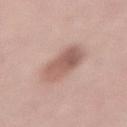biopsy_status: not biopsied; imaged during a skin examination
patient:
  sex: female
  age_approx: 40
lesion_size:
  long_diameter_mm_approx: 6.0
automated_metrics:
  vs_skin_darker_L: 12.0
  vs_skin_contrast_norm: 7.5
lighting: white-light
image:
  source: total-body photography crop
  field_of_view_mm: 15
site: lower back